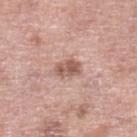Q: Was this lesion biopsied?
A: no biopsy performed (imaged during a skin exam)
Q: How was the tile lit?
A: white-light
Q: How large is the lesion?
A: about 3 mm
Q: What are the patient's age and sex?
A: male, approximately 55 years of age
Q: Lesion location?
A: the left lower leg
Q: How was this image acquired?
A: ~15 mm crop, total-body skin-cancer survey